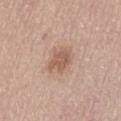Clinical impression:
The lesion was tiled from a total-body skin photograph and was not biopsied.
Image and clinical context:
Automated image analysis of the tile measured a shape eccentricity near 0.6 and two-axis asymmetry of about 0.3. The analysis additionally found a mean CIELAB color near L≈57 a*≈20 b*≈28 and a normalized lesion–skin contrast near 6.5. And it measured a within-lesion color-variation index near 2/10. The analysis additionally found a nevus-likeness score of about 25/100 and a lesion-detection confidence of about 100/100. The lesion is located on the abdomen. A close-up tile cropped from a whole-body skin photograph, about 15 mm across. The patient is a female aged around 65. Captured under white-light illumination. Approximately 3 mm at its widest.